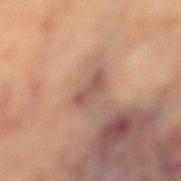follow-up: no biopsy performed (imaged during a skin exam)
lesion diameter: ~3.5 mm (longest diameter)
location: the right leg
automated metrics: a lesion color around L≈55 a*≈21 b*≈25 in CIELAB and about 9 CIELAB-L* units darker than the surrounding skin; internal color variation of about 3 on a 0–10 scale and a peripheral color-asymmetry measure near 1; an automated nevus-likeness rating near 0 out of 100 and a lesion-detection confidence of about 70/100
image: ~15 mm crop, total-body skin-cancer survey
lighting: cross-polarized
patient: female, in their 60s